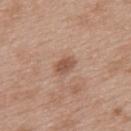  biopsy_status: not biopsied; imaged during a skin examination
  patient:
    sex: female
    age_approx: 40
  site: upper back
  lesion_size:
    long_diameter_mm_approx: 2.5
  lighting: white-light
  image:
    source: total-body photography crop
    field_of_view_mm: 15